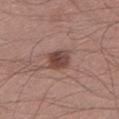Captured during whole-body skin photography for melanoma surveillance; the lesion was not biopsied. Cropped from a whole-body photographic skin survey; the tile spans about 15 mm. The lesion is located on the left lower leg. Imaged with white-light lighting. Measured at roughly 2.5 mm in maximum diameter. The total-body-photography lesion software estimated a shape eccentricity near 0.5. A male subject in their 40s.